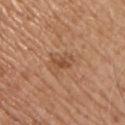Findings:
• biopsy status — total-body-photography surveillance lesion; no biopsy
• lighting — white-light
• anatomic site — the left upper arm
• image — 15 mm crop, total-body photography
• automated lesion analysis — an area of roughly 3.5 mm² and an outline eccentricity of about 0.85 (0 = round, 1 = elongated)
• subject — male, about 65 years old